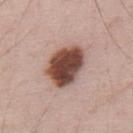acquisition: total-body-photography crop, ~15 mm field of view | subject: male, aged 68–72 | automated metrics: an area of roughly 16 mm², a shape eccentricity near 0.75, and a symmetry-axis asymmetry near 0.2; a mean CIELAB color near L≈46 a*≈21 b*≈25 and roughly 21 lightness units darker than nearby skin; a border-irregularity index near 2/10 and internal color variation of about 5 on a 0–10 scale; a classifier nevus-likeness of about 60/100 | location: the mid back.